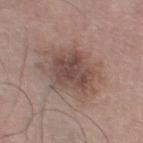Recorded during total-body skin imaging; not selected for excision or biopsy. Cropped from a whole-body photographic skin survey; the tile spans about 15 mm. Captured under white-light illumination. Approximately 7 mm at its widest. The lesion is on the left thigh. The subject is a male aged 73 to 77.